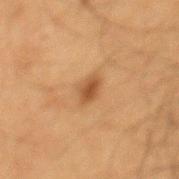Impression: The lesion was tiled from a total-body skin photograph and was not biopsied. Image and clinical context: From the mid back. This image is a 15 mm lesion crop taken from a total-body photograph. The tile uses cross-polarized illumination. The subject is a male in their mid- to late 60s. The lesion's longest dimension is about 2.5 mm.